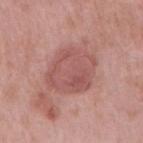biopsy_status: not biopsied; imaged during a skin examination
patient:
  sex: male
  age_approx: 55
site: right upper arm
lighting: white-light
image:
  source: total-body photography crop
  field_of_view_mm: 15